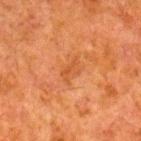Q: What is the lesion's diameter?
A: about 2.5 mm
Q: How was the tile lit?
A: cross-polarized
Q: What is the anatomic site?
A: the right lower leg
Q: What are the patient's age and sex?
A: male, aged approximately 80
Q: What is the imaging modality?
A: 15 mm crop, total-body photography